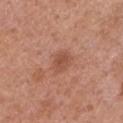Imaged during a routine full-body skin examination; the lesion was not biopsied and no histopathology is available.
Measured at roughly 2.5 mm in maximum diameter.
The subject is a female in their 50s.
A roughly 15 mm field-of-view crop from a total-body skin photograph.
On the front of the torso.
Imaged with white-light lighting.